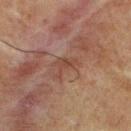Recorded during total-body skin imaging; not selected for excision or biopsy. Measured at roughly 3 mm in maximum diameter. A 15 mm crop from a total-body photograph taken for skin-cancer surveillance. Imaged with cross-polarized lighting. The lesion is on the front of the torso. A male subject, about 75 years old.